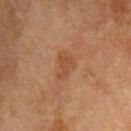follow-up — imaged on a skin check; not biopsied
site — the left arm
patient — male, in their mid- to late 60s
acquisition — ~15 mm tile from a whole-body skin photo